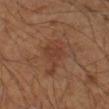Impression:
Imaged during a routine full-body skin examination; the lesion was not biopsied and no histopathology is available.
Acquisition and patient details:
This is a cross-polarized tile. From the left arm. An algorithmic analysis of the crop reported a lesion color around L≈37 a*≈21 b*≈27 in CIELAB, roughly 5 lightness units darker than nearby skin, and a normalized border contrast of about 5. The software also gave a border-irregularity index near 4.5/10, a within-lesion color-variation index near 2.5/10, and radial color variation of about 1. The software also gave a nevus-likeness score of about 10/100 and lesion-presence confidence of about 100/100. A male subject, roughly 65 years of age. A roughly 15 mm field-of-view crop from a total-body skin photograph. Longest diameter approximately 3 mm.